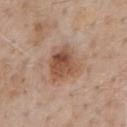| key | value |
|---|---|
| biopsy status | catalogued during a skin exam; not biopsied |
| lighting | white-light illumination |
| patient | male, aged approximately 60 |
| body site | the chest |
| image | total-body-photography crop, ~15 mm field of view |
| automated lesion analysis | a lesion area of about 13 mm², an eccentricity of roughly 0.55, and two-axis asymmetry of about 0.25; border irregularity of about 2.5 on a 0–10 scale and a color-variation rating of about 7/10 |